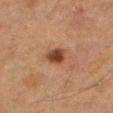Impression: The lesion was photographed on a routine skin check and not biopsied; there is no pathology result. Background: Approximately 3 mm at its widest. The total-body-photography lesion software estimated a footprint of about 6 mm² and a shape-asymmetry score of about 0.2 (0 = symmetric). And it measured a mean CIELAB color near L≈35 a*≈19 b*≈27, a lesion–skin lightness drop of about 11, and a normalized lesion–skin contrast near 10. The analysis additionally found a border-irregularity rating of about 2/10, a color-variation rating of about 4.5/10, and a peripheral color-asymmetry measure near 1.5. A region of skin cropped from a whole-body photographic capture, roughly 15 mm wide. The lesion is on the right thigh. A male patient, aged approximately 75.